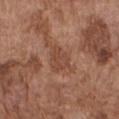Findings:
– notes: catalogued during a skin exam; not biopsied
– body site: the front of the torso
– automated metrics: a lesion area of about 5.5 mm² and a shape eccentricity near 0.75; a lesion–skin lightness drop of about 7 and a normalized border contrast of about 5.5; a border-irregularity rating of about 4/10, a color-variation rating of about 2/10, and peripheral color asymmetry of about 0.5
– imaging modality: 15 mm crop, total-body photography
– subject: male, about 75 years old